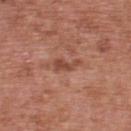Assessment:
Imaged during a routine full-body skin examination; the lesion was not biopsied and no histopathology is available.
Acquisition and patient details:
A region of skin cropped from a whole-body photographic capture, roughly 15 mm wide. The lesion-visualizer software estimated a border-irregularity rating of about 6/10, internal color variation of about 0.5 on a 0–10 scale, and a peripheral color-asymmetry measure near 0. On the upper back. A male patient in their 70s. The recorded lesion diameter is about 3.5 mm.